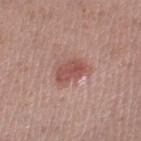follow-up: total-body-photography surveillance lesion; no biopsy
subject: female, aged 53–57
image: ~15 mm crop, total-body skin-cancer survey
location: the right lower leg
lighting: white-light
lesion size: about 4 mm